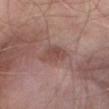Imaged during a routine full-body skin examination; the lesion was not biopsied and no histopathology is available. The lesion is located on the abdomen. The patient is a male aged 48 to 52. A 15 mm close-up extracted from a 3D total-body photography capture. The lesion's longest dimension is about 3.5 mm.